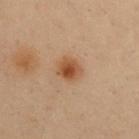{"biopsy_status": "not biopsied; imaged during a skin examination", "site": "chest", "lesion_size": {"long_diameter_mm_approx": 3.0}, "patient": {"sex": "female", "age_approx": 50}, "image": {"source": "total-body photography crop", "field_of_view_mm": 15}}